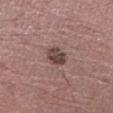Recorded during total-body skin imaging; not selected for excision or biopsy. A male subject, aged approximately 35. This is a white-light tile. The lesion is located on the left lower leg. An algorithmic analysis of the crop reported an area of roughly 5 mm², an outline eccentricity of about 0.6 (0 = round, 1 = elongated), and a symmetry-axis asymmetry near 0.2. A 15 mm crop from a total-body photograph taken for skin-cancer surveillance.